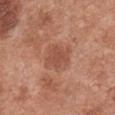This lesion was catalogued during total-body skin photography and was not selected for biopsy. Automated tile analysis of the lesion measured a footprint of about 6 mm², a shape eccentricity near 0.55, and two-axis asymmetry of about 0.2. And it measured an average lesion color of about L≈50 a*≈25 b*≈30 (CIELAB), about 8 CIELAB-L* units darker than the surrounding skin, and a normalized border contrast of about 5.5. About 3 mm across. Located on the chest. Captured under white-light illumination. A lesion tile, about 15 mm wide, cut from a 3D total-body photograph. The subject is a female about 65 years old.